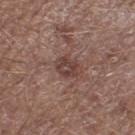Q: Was this lesion biopsied?
A: catalogued during a skin exam; not biopsied
Q: Automated lesion metrics?
A: a lesion area of about 6 mm², a shape eccentricity near 0.55, and a shape-asymmetry score of about 0.25 (0 = symmetric); a lesion color around L≈41 a*≈19 b*≈21 in CIELAB, a lesion–skin lightness drop of about 9, and a lesion-to-skin contrast of about 7 (normalized; higher = more distinct)
Q: What is the imaging modality?
A: ~15 mm tile from a whole-body skin photo
Q: Lesion location?
A: the right lower leg
Q: Lesion size?
A: ≈3 mm
Q: Illumination type?
A: white-light illumination
Q: Patient demographics?
A: male, aged 68–72